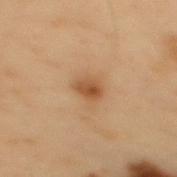biopsy_status: not biopsied; imaged during a skin examination
lighting: cross-polarized
patient:
  sex: male
  age_approx: 55
site: back
automated_metrics:
  color_variation_0_10: 4.0
  peripheral_color_asymmetry: 1.5
lesion_size:
  long_diameter_mm_approx: 2.5
image:
  source: total-body photography crop
  field_of_view_mm: 15